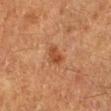– acquisition — ~15 mm crop, total-body skin-cancer survey
– subject — female, roughly 50 years of age
– anatomic site — the right lower leg
– lesion diameter — ~3 mm (longest diameter)
– automated metrics — a border-irregularity rating of about 3/10, a color-variation rating of about 3/10, and radial color variation of about 1
– lighting — cross-polarized illumination